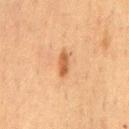Clinical impression:
The lesion was photographed on a routine skin check and not biopsied; there is no pathology result.
Background:
A 15 mm close-up extracted from a 3D total-body photography capture. The total-body-photography lesion software estimated a footprint of about 3.5 mm², an eccentricity of roughly 0.9, and two-axis asymmetry of about 0.15. The analysis additionally found a border-irregularity index near 2/10, a within-lesion color-variation index near 2/10, and peripheral color asymmetry of about 0.5. And it measured a lesion-detection confidence of about 100/100. The lesion's longest dimension is about 3 mm. On the chest. The tile uses cross-polarized illumination. The patient is a male aged 48 to 52.